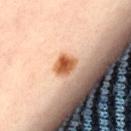biopsy status = no biopsy performed (imaged during a skin exam) | image source = total-body-photography crop, ~15 mm field of view | illumination = cross-polarized illumination | location = the left thigh | automated lesion analysis = a lesion area of about 5 mm² and an eccentricity of roughly 0.4; an automated nevus-likeness rating near 100 out of 100 and a lesion-detection confidence of about 100/100 | lesion size = ~2.5 mm (longest diameter) | patient = male, in their mid-60s.